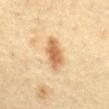Q: Was this lesion biopsied?
A: imaged on a skin check; not biopsied
Q: What is the imaging modality?
A: 15 mm crop, total-body photography
Q: What is the lesion's diameter?
A: about 4.5 mm
Q: Patient demographics?
A: female, about 55 years old
Q: How was the tile lit?
A: cross-polarized illumination
Q: What is the anatomic site?
A: the abdomen